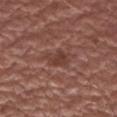Notes:
• notes · catalogued during a skin exam; not biopsied
• patient · male, aged approximately 60
• illumination · white-light
• image source · total-body-photography crop, ~15 mm field of view
• anatomic site · the right upper arm